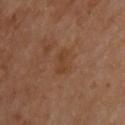Assessment:
Imaged during a routine full-body skin examination; the lesion was not biopsied and no histopathology is available.
Background:
The subject is a male approximately 65 years of age. The recorded lesion diameter is about 3 mm. The total-body-photography lesion software estimated border irregularity of about 3.5 on a 0–10 scale, internal color variation of about 1.5 on a 0–10 scale, and radial color variation of about 0.5. It also reported lesion-presence confidence of about 100/100. A close-up tile cropped from a whole-body skin photograph, about 15 mm across. The lesion is on the upper back. The tile uses cross-polarized illumination.